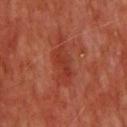biopsy status: imaged on a skin check; not biopsied | image source: ~15 mm crop, total-body skin-cancer survey | subject: male, approximately 60 years of age | body site: the head or neck | lighting: cross-polarized | lesion diameter: about 4.5 mm.